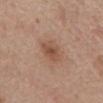A male subject aged around 80.
This image is a 15 mm lesion crop taken from a total-body photograph.
The total-body-photography lesion software estimated an area of roughly 7 mm², an eccentricity of roughly 0.75, and two-axis asymmetry of about 0.2. It also reported a lesion color around L≈51 a*≈20 b*≈30 in CIELAB, about 9 CIELAB-L* units darker than the surrounding skin, and a lesion-to-skin contrast of about 6.5 (normalized; higher = more distinct). The analysis additionally found a nevus-likeness score of about 25/100 and lesion-presence confidence of about 100/100.
On the abdomen.
The tile uses white-light illumination.